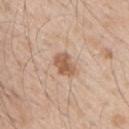Q: How was this image acquired?
A: total-body-photography crop, ~15 mm field of view
Q: How was the tile lit?
A: white-light illumination
Q: What is the anatomic site?
A: the right upper arm
Q: What did automated image analysis measure?
A: a shape eccentricity near 0.6 and two-axis asymmetry of about 0.2; a lesion color around L≈58 a*≈20 b*≈32 in CIELAB and roughly 12 lightness units darker than nearby skin
Q: How large is the lesion?
A: ≈3 mm
Q: Who is the patient?
A: male, about 50 years old